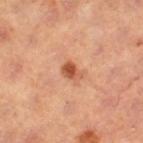Clinical impression: Recorded during total-body skin imaging; not selected for excision or biopsy. Image and clinical context: The tile uses cross-polarized illumination. The total-body-photography lesion software estimated a shape eccentricity near 0.75 and a shape-asymmetry score of about 0.25 (0 = symmetric). The software also gave a border-irregularity rating of about 2/10, a color-variation rating of about 3/10, and a peripheral color-asymmetry measure near 1. The analysis additionally found a classifier nevus-likeness of about 90/100 and lesion-presence confidence of about 100/100. A 15 mm close-up tile from a total-body photography series done for melanoma screening. Longest diameter approximately 2.5 mm. The subject is a female aged around 40. From the right thigh.